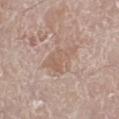Clinical impression: Part of a total-body skin-imaging series; this lesion was reviewed on a skin check and was not flagged for biopsy. Context: Captured under white-light illumination. A 15 mm close-up extracted from a 3D total-body photography capture. About 4.5 mm across. A male subject approximately 80 years of age. From the left leg. Automated image analysis of the tile measured an average lesion color of about L≈59 a*≈17 b*≈26 (CIELAB), a lesion–skin lightness drop of about 7, and a normalized border contrast of about 5. It also reported a border-irregularity rating of about 5.5/10, internal color variation of about 2 on a 0–10 scale, and peripheral color asymmetry of about 0.5. And it measured a detector confidence of about 100 out of 100 that the crop contains a lesion.